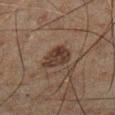Notes:
• biopsy status — no biopsy performed (imaged during a skin exam)
• site — the left lower leg
• patient — male, in their 60s
• tile lighting — cross-polarized
• automated lesion analysis — a lesion color around L≈26 a*≈13 b*≈19 in CIELAB, about 8 CIELAB-L* units darker than the surrounding skin, and a lesion-to-skin contrast of about 9 (normalized; higher = more distinct); a nevus-likeness score of about 50/100 and a lesion-detection confidence of about 100/100
• acquisition — ~15 mm crop, total-body skin-cancer survey
• lesion diameter — ~3.5 mm (longest diameter)